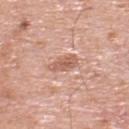follow-up = no biopsy performed (imaged during a skin exam) | TBP lesion metrics = a footprint of about 4.5 mm², an eccentricity of roughly 0.9, and two-axis asymmetry of about 0.25; a mean CIELAB color near L≈59 a*≈23 b*≈29 and a normalized lesion–skin contrast near 6.5; a border-irregularity rating of about 2.5/10, internal color variation of about 3 on a 0–10 scale, and radial color variation of about 1 | illumination = white-light illumination | site = the upper back | acquisition = ~15 mm tile from a whole-body skin photo | subject = male, aged 78–82 | diameter = about 3.5 mm.